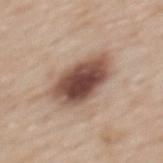Impression: Recorded during total-body skin imaging; not selected for excision or biopsy. Context: The patient is a female aged 48 to 52. A 15 mm close-up tile from a total-body photography series done for melanoma screening. The lesion is on the upper back.